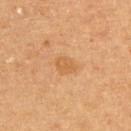Notes:
- follow-up · imaged on a skin check; not biopsied
- subject · female, aged approximately 60
- acquisition · total-body-photography crop, ~15 mm field of view
- lighting · cross-polarized
- diameter · about 2.5 mm
- body site · the upper back